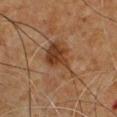biopsy status: no biopsy performed (imaged during a skin exam); image: 15 mm crop, total-body photography; lesion size: ~6 mm (longest diameter); patient: male, roughly 60 years of age; body site: the chest.